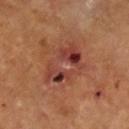<tbp_lesion>
<biopsy_status>not biopsied; imaged during a skin examination</biopsy_status>
<lesion_size>
  <long_diameter_mm_approx>6.0</long_diameter_mm_approx>
</lesion_size>
<image>
  <source>total-body photography crop</source>
  <field_of_view_mm>15</field_of_view_mm>
</image>
<automated_metrics>
  <cielab_L>40</cielab_L>
  <cielab_a>26</cielab_a>
  <cielab_b>27</cielab_b>
  <vs_skin_contrast_norm>8.0</vs_skin_contrast_norm>
  <color_variation_0_10>10.0</color_variation_0_10>
  <peripheral_color_asymmetry>4.0</peripheral_color_asymmetry>
</automated_metrics>
<site>left leg</site>
<lighting>cross-polarized</lighting>
<patient>
  <sex>male</sex>
  <age_approx>65</age_approx>
</patient>
</tbp_lesion>